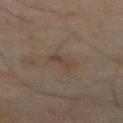workup: total-body-photography surveillance lesion; no biopsy
location: the abdomen
lighting: cross-polarized
subject: male, about 70 years old
image: ~15 mm crop, total-body skin-cancer survey